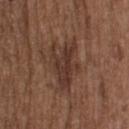Assessment: Imaged during a routine full-body skin examination; the lesion was not biopsied and no histopathology is available. Acquisition and patient details: A 15 mm close-up tile from a total-body photography series done for melanoma screening. The subject is a male approximately 50 years of age. On the back.